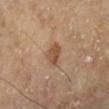Part of a total-body skin-imaging series; this lesion was reviewed on a skin check and was not flagged for biopsy.
A male patient, aged 63 to 67.
A roughly 15 mm field-of-view crop from a total-body skin photograph.
From the left lower leg.